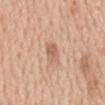Impression:
Recorded during total-body skin imaging; not selected for excision or biopsy.
Context:
A male subject, in their 60s. A 15 mm crop from a total-body photograph taken for skin-cancer surveillance. Approximately 3 mm at its widest. From the mid back.